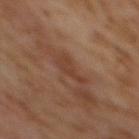workup: total-body-photography surveillance lesion; no biopsy | anatomic site: the back | acquisition: 15 mm crop, total-body photography | patient: female, aged approximately 60.